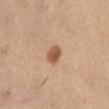biopsy status — no biopsy performed (imaged during a skin exam); subject — female, about 35 years old; diameter — ~2.5 mm (longest diameter); image source — total-body-photography crop, ~15 mm field of view; anatomic site — the left lower leg.